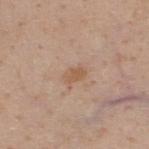workup: imaged on a skin check; not biopsied
anatomic site: the upper back
image-analysis metrics: a lesion area of about 3.5 mm², an outline eccentricity of about 0.8 (0 = round, 1 = elongated), and a symmetry-axis asymmetry near 0.25; an average lesion color of about L≈56 a*≈18 b*≈31 (CIELAB), a lesion–skin lightness drop of about 7, and a lesion-to-skin contrast of about 6 (normalized; higher = more distinct)
subject: male, in their 40s
tile lighting: white-light illumination
image: total-body-photography crop, ~15 mm field of view